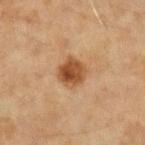This lesion was catalogued during total-body skin photography and was not selected for biopsy. About 3 mm across. Imaged with cross-polarized lighting. Automated tile analysis of the lesion measured an area of roughly 8 mm², an outline eccentricity of about 0.4 (0 = round, 1 = elongated), and a shape-asymmetry score of about 0.2 (0 = symmetric). And it measured border irregularity of about 2 on a 0–10 scale and a peripheral color-asymmetry measure near 2. The subject is a female approximately 40 years of age. From the left forearm. A 15 mm close-up extracted from a 3D total-body photography capture.